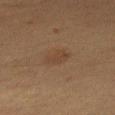notes=total-body-photography surveillance lesion; no biopsy
site=the right thigh
size=about 3 mm
patient=female, approximately 55 years of age
acquisition=15 mm crop, total-body photography
illumination=cross-polarized
image-analysis metrics=a lesion area of about 5 mm², a shape eccentricity near 0.8, and two-axis asymmetry of about 0.2; a lesion color around L≈33 a*≈14 b*≈24 in CIELAB, roughly 5 lightness units darker than nearby skin, and a normalized border contrast of about 5; a border-irregularity index near 2/10, internal color variation of about 1.5 on a 0–10 scale, and radial color variation of about 0.5